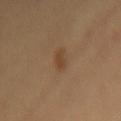About 3 mm across. This is a cross-polarized tile. An algorithmic analysis of the crop reported a footprint of about 3 mm², an outline eccentricity of about 0.95 (0 = round, 1 = elongated), and a symmetry-axis asymmetry near 0.3. And it measured roughly 7 lightness units darker than nearby skin and a normalized lesion–skin contrast near 6.5. The software also gave a classifier nevus-likeness of about 55/100 and lesion-presence confidence of about 100/100. On the mid back. A region of skin cropped from a whole-body photographic capture, roughly 15 mm wide. The patient is a female roughly 60 years of age.